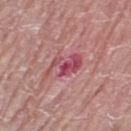Recorded during total-body skin imaging; not selected for excision or biopsy. Longest diameter approximately 4.5 mm. A female patient aged 58 to 62. A roughly 15 mm field-of-view crop from a total-body skin photograph. An algorithmic analysis of the crop reported an area of roughly 8 mm², an outline eccentricity of about 0.85 (0 = round, 1 = elongated), and a symmetry-axis asymmetry near 0.5. And it measured a lesion color around L≈50 a*≈33 b*≈18 in CIELAB. From the right thigh.